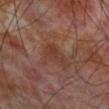Clinical impression:
No biopsy was performed on this lesion — it was imaged during a full skin examination and was not determined to be concerning.
Clinical summary:
On the right forearm. A roughly 15 mm field-of-view crop from a total-body skin photograph. Imaged with cross-polarized lighting. A male patient aged approximately 70.